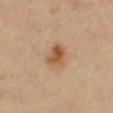This lesion was catalogued during total-body skin photography and was not selected for biopsy. The lesion is on the right thigh. A female patient, approximately 40 years of age. A 15 mm close-up tile from a total-body photography series done for melanoma screening. Imaged with cross-polarized lighting. Measured at roughly 3 mm in maximum diameter.